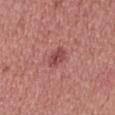<tbp_lesion>
<biopsy_status>not biopsied; imaged during a skin examination</biopsy_status>
</tbp_lesion>